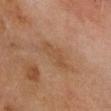| field | value |
|---|---|
| follow-up | catalogued during a skin exam; not biopsied |
| location | the head or neck |
| subject | male, about 60 years old |
| lighting | cross-polarized |
| imaging modality | total-body-photography crop, ~15 mm field of view |
| diameter | ≈4 mm |